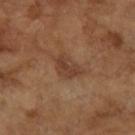The lesion was tiled from a total-body skin photograph and was not biopsied.